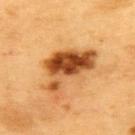follow-up = no biopsy performed (imaged during a skin exam) | image = ~15 mm tile from a whole-body skin photo | illumination = cross-polarized | subject = male, aged 58 to 62 | anatomic site = the upper back | lesion size = about 7 mm.